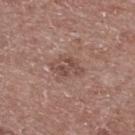A male patient, in their 60s. Cropped from a whole-body photographic skin survey; the tile spans about 15 mm. On the upper back. The recorded lesion diameter is about 3.5 mm. Automated tile analysis of the lesion measured a border-irregularity index near 3.5/10 and a within-lesion color-variation index near 2.5/10. The software also gave a classifier nevus-likeness of about 0/100 and a detector confidence of about 100 out of 100 that the crop contains a lesion.